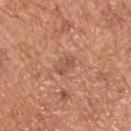This lesion was catalogued during total-body skin photography and was not selected for biopsy. The tile uses white-light illumination. A male patient, in their mid-60s. A region of skin cropped from a whole-body photographic capture, roughly 15 mm wide. Measured at roughly 2.5 mm in maximum diameter. Automated tile analysis of the lesion measured an outline eccentricity of about 0.65 (0 = round, 1 = elongated). On the upper back.